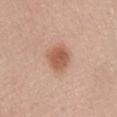Q: Was this lesion biopsied?
A: total-body-photography surveillance lesion; no biopsy
Q: What are the patient's age and sex?
A: female, aged approximately 50
Q: How was this image acquired?
A: total-body-photography crop, ~15 mm field of view
Q: Where on the body is the lesion?
A: the head or neck